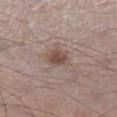{
  "biopsy_status": "not biopsied; imaged during a skin examination",
  "image": {
    "source": "total-body photography crop",
    "field_of_view_mm": 15
  },
  "lighting": "white-light",
  "site": "left lower leg",
  "lesion_size": {
    "long_diameter_mm_approx": 3.0
  },
  "patient": {
    "sex": "male",
    "age_approx": 55
  }
}